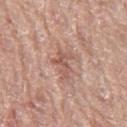Q: Was a biopsy performed?
A: total-body-photography surveillance lesion; no biopsy
Q: What kind of image is this?
A: total-body-photography crop, ~15 mm field of view
Q: What are the patient's age and sex?
A: female, about 60 years old
Q: What is the anatomic site?
A: the leg
Q: What is the lesion's diameter?
A: about 3.5 mm
Q: Illumination type?
A: white-light illumination